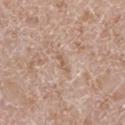<record>
<patient>
  <sex>male</sex>
  <age_approx>60</age_approx>
</patient>
<site>left lower leg</site>
<image>
  <source>total-body photography crop</source>
  <field_of_view_mm>15</field_of_view_mm>
</image>
<lighting>white-light</lighting>
<lesion_size>
  <long_diameter_mm_approx>2.5</long_diameter_mm_approx>
</lesion_size>
</record>